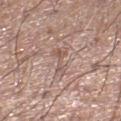Q: What are the patient's age and sex?
A: male, aged around 20
Q: Automated lesion metrics?
A: a border-irregularity index near 6/10, internal color variation of about 3.5 on a 0–10 scale, and a peripheral color-asymmetry measure near 1
Q: How was this image acquired?
A: 15 mm crop, total-body photography
Q: Where on the body is the lesion?
A: the leg
Q: How large is the lesion?
A: ~4 mm (longest diameter)
Q: How was the tile lit?
A: white-light